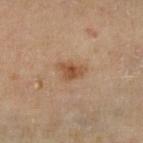{
  "biopsy_status": "not biopsied; imaged during a skin examination",
  "image": {
    "source": "total-body photography crop",
    "field_of_view_mm": 15
  },
  "lesion_size": {
    "long_diameter_mm_approx": 3.0
  },
  "patient": {
    "sex": "female",
    "age_approx": 60
  },
  "site": "left lower leg",
  "automated_metrics": {
    "border_irregularity_0_10": 3.5,
    "color_variation_0_10": 2.5,
    "peripheral_color_asymmetry": 0.5
  }
}